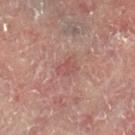No biopsy was performed on this lesion — it was imaged during a full skin examination and was not determined to be concerning. The tile uses cross-polarized illumination. The total-body-photography lesion software estimated a footprint of about 4 mm², an outline eccentricity of about 0.75 (0 = round, 1 = elongated), and two-axis asymmetry of about 0.3. The analysis additionally found a mean CIELAB color near L≈46 a*≈22 b*≈21, about 6 CIELAB-L* units darker than the surrounding skin, and a lesion-to-skin contrast of about 5 (normalized; higher = more distinct). The lesion is located on the right lower leg. A 15 mm close-up tile from a total-body photography series done for melanoma screening. The lesion's longest dimension is about 2.5 mm. A male subject, approximately 60 years of age.